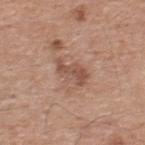Captured during whole-body skin photography for melanoma surveillance; the lesion was not biopsied. A male patient, approximately 55 years of age. A lesion tile, about 15 mm wide, cut from a 3D total-body photograph. From the upper back.